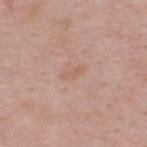The lesion was tiled from a total-body skin photograph and was not biopsied. Imaged with white-light lighting. The lesion's longest dimension is about 2.5 mm. This image is a 15 mm lesion crop taken from a total-body photograph. The patient is a male aged around 50. The lesion is on the upper back. The total-body-photography lesion software estimated a lesion area of about 2 mm², an eccentricity of roughly 0.95, and a symmetry-axis asymmetry near 0.4. The analysis additionally found a mean CIELAB color near L≈60 a*≈20 b*≈29, about 5 CIELAB-L* units darker than the surrounding skin, and a lesion-to-skin contrast of about 5 (normalized; higher = more distinct). It also reported border irregularity of about 4.5 on a 0–10 scale and peripheral color asymmetry of about 0. The software also gave a lesion-detection confidence of about 100/100.